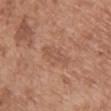{
  "biopsy_status": "not biopsied; imaged during a skin examination",
  "site": "back",
  "image": {
    "source": "total-body photography crop",
    "field_of_view_mm": 15
  },
  "patient": {
    "sex": "female",
    "age_approx": 75
  },
  "lighting": "white-light",
  "lesion_size": {
    "long_diameter_mm_approx": 4.5
  },
  "automated_metrics": {
    "area_mm2_approx": 4.0,
    "eccentricity": 0.95,
    "shape_asymmetry": 0.3,
    "border_irregularity_0_10": 5.0,
    "color_variation_0_10": 0.0,
    "peripheral_color_asymmetry": 0.0,
    "nevus_likeness_0_100": 0
  }
}